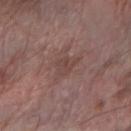Captured under white-light illumination.
A male patient aged 63 to 67.
Cropped from a whole-body photographic skin survey; the tile spans about 15 mm.
The lesion is located on the arm.